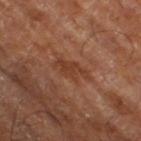workup = catalogued during a skin exam; not biopsied | body site = the right thigh | TBP lesion metrics = about 6 CIELAB-L* units darker than the surrounding skin and a normalized border contrast of about 6; border irregularity of about 4 on a 0–10 scale, a within-lesion color-variation index near 2.5/10, and a peripheral color-asymmetry measure near 1; a classifier nevus-likeness of about 5/100 and a lesion-detection confidence of about 95/100 | patient = approximately 65 years of age | image = total-body-photography crop, ~15 mm field of view | lesion diameter = about 4.5 mm.